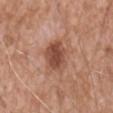acquisition=15 mm crop, total-body photography
lesion size=≈4.5 mm
subject=male, approximately 60 years of age
automated metrics=a lesion color around L≈49 a*≈23 b*≈29 in CIELAB, a lesion–skin lightness drop of about 12, and a normalized border contrast of about 9; a border-irregularity index near 2.5/10, internal color variation of about 3.5 on a 0–10 scale, and radial color variation of about 1; a nevus-likeness score of about 40/100 and lesion-presence confidence of about 100/100
site=the abdomen